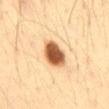{
  "biopsy_status": "not biopsied; imaged during a skin examination",
  "automated_metrics": {
    "area_mm2_approx": 8.5,
    "eccentricity": 0.65,
    "shape_asymmetry": 0.15,
    "cielab_L": 53,
    "cielab_a": 22,
    "cielab_b": 37,
    "vs_skin_darker_L": 22.0,
    "vs_skin_contrast_norm": 13.5,
    "border_irregularity_0_10": 1.5,
    "color_variation_0_10": 4.5,
    "peripheral_color_asymmetry": 1.0
  },
  "image": {
    "source": "total-body photography crop",
    "field_of_view_mm": 15
  },
  "lesion_size": {
    "long_diameter_mm_approx": 3.5
  },
  "patient": {
    "sex": "male",
    "age_approx": 35
  },
  "site": "abdomen",
  "lighting": "cross-polarized"
}